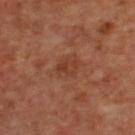Part of a total-body skin-imaging series; this lesion was reviewed on a skin check and was not flagged for biopsy.
The total-body-photography lesion software estimated an area of roughly 4 mm², a shape eccentricity near 0.75, and a shape-asymmetry score of about 0.25 (0 = symmetric). The software also gave a lesion color around L≈37 a*≈24 b*≈30 in CIELAB, a lesion–skin lightness drop of about 7, and a lesion-to-skin contrast of about 6 (normalized; higher = more distinct). It also reported a classifier nevus-likeness of about 0/100 and a detector confidence of about 100 out of 100 that the crop contains a lesion.
This is a cross-polarized tile.
The lesion is on the upper back.
The subject is a female roughly 45 years of age.
Approximately 2.5 mm at its widest.
A 15 mm close-up tile from a total-body photography series done for melanoma screening.